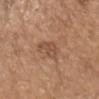Case summary:
* follow-up — catalogued during a skin exam; not biopsied
* lighting — white-light
* automated lesion analysis — border irregularity of about 4 on a 0–10 scale, a color-variation rating of about 2/10, and radial color variation of about 0.5; a classifier nevus-likeness of about 5/100 and a detector confidence of about 100 out of 100 that the crop contains a lesion
* patient — male, roughly 70 years of age
* lesion diameter — about 3.5 mm
* imaging modality — ~15 mm tile from a whole-body skin photo
* anatomic site — the head or neck